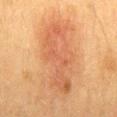The lesion was photographed on a routine skin check and not biopsied; there is no pathology result. This image is a 15 mm lesion crop taken from a total-body photograph. The patient is a male aged 33 to 37. The lesion is on the mid back. The lesion's longest dimension is about 9.5 mm. Captured under cross-polarized illumination.